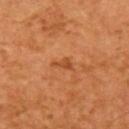Clinical summary: From the left upper arm. A subject roughly 65 years of age. An algorithmic analysis of the crop reported a mean CIELAB color near L≈49 a*≈28 b*≈41 and a lesion–skin lightness drop of about 8. The software also gave a border-irregularity rating of about 4/10, a color-variation rating of about 1/10, and radial color variation of about 0.5. The software also gave a classifier nevus-likeness of about 0/100 and lesion-presence confidence of about 100/100. About 2.5 mm across. A region of skin cropped from a whole-body photographic capture, roughly 15 mm wide.